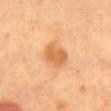  lighting: cross-polarized
  image:
    source: total-body photography crop
    field_of_view_mm: 15
  site: abdomen
  lesion_size:
    long_diameter_mm_approx: 3.5
  patient:
    sex: female
    age_approx: 60